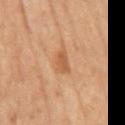follow-up: imaged on a skin check; not biopsied
patient: male, aged around 70
anatomic site: the mid back
image source: ~15 mm tile from a whole-body skin photo